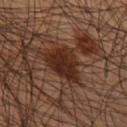Assessment: The lesion was photographed on a routine skin check and not biopsied; there is no pathology result. Clinical summary: The patient is a male in their mid- to late 40s. A region of skin cropped from a whole-body photographic capture, roughly 15 mm wide. This is a cross-polarized tile. Located on the right lower leg.